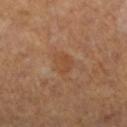Clinical impression: Recorded during total-body skin imaging; not selected for excision or biopsy. Background: A 15 mm crop from a total-body photograph taken for skin-cancer surveillance. The tile uses cross-polarized illumination. The patient is a female aged approximately 65. The lesion is on the left lower leg. The recorded lesion diameter is about 3 mm.